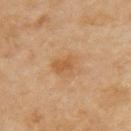Q: How was the tile lit?
A: cross-polarized
Q: What kind of image is this?
A: ~15 mm tile from a whole-body skin photo
Q: What is the lesion's diameter?
A: ≈3 mm
Q: Automated lesion metrics?
A: a footprint of about 6 mm², an eccentricity of roughly 0.65, and two-axis asymmetry of about 0.3; an average lesion color of about L≈52 a*≈19 b*≈36 (CIELAB), roughly 7 lightness units darker than nearby skin, and a normalized border contrast of about 6; border irregularity of about 2.5 on a 0–10 scale and a within-lesion color-variation index near 3.5/10; a nevus-likeness score of about 10/100 and lesion-presence confidence of about 100/100
Q: Where on the body is the lesion?
A: the left upper arm
Q: Who is the patient?
A: female, approximately 60 years of age